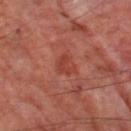Q: Was this lesion biopsied?
A: imaged on a skin check; not biopsied